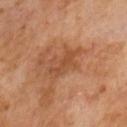<case>
<biopsy_status>not biopsied; imaged during a skin examination</biopsy_status>
<image>
  <source>total-body photography crop</source>
  <field_of_view_mm>15</field_of_view_mm>
</image>
<patient>
  <sex>male</sex>
  <age_approx>65</age_approx>
</patient>
<automated_metrics>
  <cielab_L>48</cielab_L>
  <cielab_a>23</cielab_a>
  <cielab_b>35</cielab_b>
  <vs_skin_contrast_norm>6.0</vs_skin_contrast_norm>
  <border_irregularity_0_10>4.5</border_irregularity_0_10>
  <color_variation_0_10>2.5</color_variation_0_10>
  <peripheral_color_asymmetry>1.0</peripheral_color_asymmetry>
  <nevus_likeness_0_100>0</nevus_likeness_0_100>
  <lesion_detection_confidence_0_100>100</lesion_detection_confidence_0_100>
</automated_metrics>
<lesion_size>
  <long_diameter_mm_approx>4.5</long_diameter_mm_approx>
</lesion_size>
<lighting>cross-polarized</lighting>
</case>